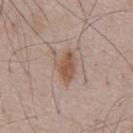The lesion was tiled from a total-body skin photograph and was not biopsied. About 4 mm across. A male patient about 55 years old. A 15 mm close-up extracted from a 3D total-body photography capture.